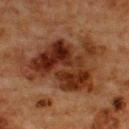The lesion was photographed on a routine skin check and not biopsied; there is no pathology result.
Longest diameter approximately 11 mm.
A 15 mm close-up extracted from a 3D total-body photography capture.
Captured under cross-polarized illumination.
The subject is a male in their mid- to late 60s.
The lesion is located on the upper back.